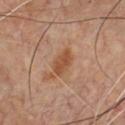Clinical impression: No biopsy was performed on this lesion — it was imaged during a full skin examination and was not determined to be concerning. Context: Imaged with cross-polarized lighting. A lesion tile, about 15 mm wide, cut from a 3D total-body photograph. The lesion is on the chest. The subject is a male roughly 60 years of age.